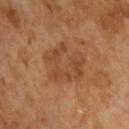The lesion was photographed on a routine skin check and not biopsied; there is no pathology result.
Cropped from a whole-body photographic skin survey; the tile spans about 15 mm.
Longest diameter approximately 5 mm.
A male subject aged 63–67.
An algorithmic analysis of the crop reported a mean CIELAB color near L≈44 a*≈21 b*≈33, a lesion–skin lightness drop of about 7, and a lesion-to-skin contrast of about 5.5 (normalized; higher = more distinct). The analysis additionally found a nevus-likeness score of about 0/100 and a lesion-detection confidence of about 100/100.